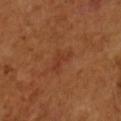Q: Was a biopsy performed?
A: catalogued during a skin exam; not biopsied
Q: Lesion location?
A: the right forearm
Q: What are the patient's age and sex?
A: female, roughly 55 years of age
Q: What is the imaging modality?
A: 15 mm crop, total-body photography